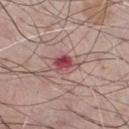The lesion was tiled from a total-body skin photograph and was not biopsied.
The lesion is on the chest.
A male subject aged 63 to 67.
A close-up tile cropped from a whole-body skin photograph, about 15 mm across.